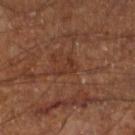Findings:
• notes · imaged on a skin check; not biopsied
• subject · male, aged approximately 60
• diameter · ≈3 mm
• anatomic site · the left lower leg
• automated lesion analysis · a lesion color around L≈33 a*≈21 b*≈27 in CIELAB and about 5 CIELAB-L* units darker than the surrounding skin; border irregularity of about 7 on a 0–10 scale, a within-lesion color-variation index near 0.5/10, and a peripheral color-asymmetry measure near 0
• image source · ~15 mm tile from a whole-body skin photo